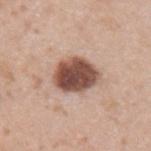{
  "biopsy_status": "not biopsied; imaged during a skin examination",
  "lighting": "white-light",
  "site": "left upper arm",
  "patient": {
    "sex": "male",
    "age_approx": 35
  },
  "image": {
    "source": "total-body photography crop",
    "field_of_view_mm": 15
  },
  "lesion_size": {
    "long_diameter_mm_approx": 5.0
  }
}